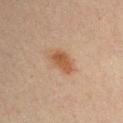automated lesion analysis: a lesion area of about 6.5 mm² and a shape-asymmetry score of about 0.3 (0 = symmetric); a mean CIELAB color near L≈44 a*≈18 b*≈29, a lesion–skin lightness drop of about 8, and a lesion-to-skin contrast of about 8 (normalized; higher = more distinct); a classifier nevus-likeness of about 95/100 and lesion-presence confidence of about 100/100
site: the left upper arm
illumination: cross-polarized illumination
subject: male, roughly 30 years of age
size: about 3.5 mm
imaging modality: total-body-photography crop, ~15 mm field of view